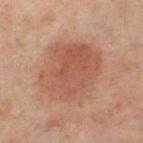Clinical impression: Part of a total-body skin-imaging series; this lesion was reviewed on a skin check and was not flagged for biopsy. Image and clinical context: The patient is a female about 50 years old. The lesion is on the right upper arm. This is a cross-polarized tile. A lesion tile, about 15 mm wide, cut from a 3D total-body photograph.